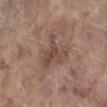Recorded during total-body skin imaging; not selected for excision or biopsy. Cropped from a total-body skin-imaging series; the visible field is about 15 mm. Located on the right lower leg. A female subject aged 83 or over.